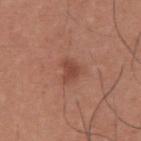Captured during whole-body skin photography for melanoma surveillance; the lesion was not biopsied. The lesion-visualizer software estimated an area of roughly 4.5 mm² and an eccentricity of roughly 0.5. The analysis additionally found about 9 CIELAB-L* units darker than the surrounding skin and a lesion-to-skin contrast of about 7 (normalized; higher = more distinct). A male subject aged 33 to 37. Captured under white-light illumination. A 15 mm close-up tile from a total-body photography series done for melanoma screening. About 2.5 mm across. The lesion is located on the upper back.